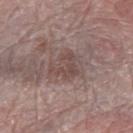notes=no biopsy performed (imaged during a skin exam)
subject=female, roughly 65 years of age
image=15 mm crop, total-body photography
lighting=white-light
lesion size=≈3.5 mm
site=the right forearm